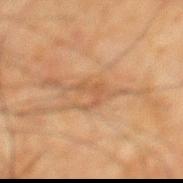location: the back
tile lighting: cross-polarized illumination
lesion diameter: about 3 mm
image: ~15 mm crop, total-body skin-cancer survey
automated metrics: a lesion color around L≈44 a*≈18 b*≈30 in CIELAB and a lesion–skin lightness drop of about 6
patient: male, roughly 65 years of age